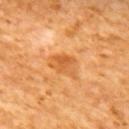No biopsy was performed on this lesion — it was imaged during a full skin examination and was not determined to be concerning.
The total-body-photography lesion software estimated a lesion area of about 7.5 mm², a shape eccentricity near 0.75, and a shape-asymmetry score of about 0.35 (0 = symmetric). And it measured a border-irregularity index near 3.5/10, internal color variation of about 4 on a 0–10 scale, and peripheral color asymmetry of about 1.5.
The lesion is located on the upper back.
Imaged with cross-polarized lighting.
A 15 mm close-up extracted from a 3D total-body photography capture.
Longest diameter approximately 4 mm.
A female patient, aged 63 to 67.